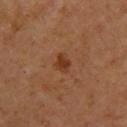workup: imaged on a skin check; not biopsied | imaging modality: total-body-photography crop, ~15 mm field of view | body site: the upper back | subject: female, approximately 40 years of age.